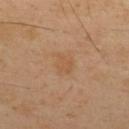Notes:
• lesion size: about 2.5 mm
• subject: male, aged around 50
• imaging modality: total-body-photography crop, ~15 mm field of view
• location: the upper back
• tile lighting: cross-polarized illumination
• image-analysis metrics: a footprint of about 5 mm², an eccentricity of roughly 0.65, and a shape-asymmetry score of about 0.3 (0 = symmetric); a lesion color around L≈54 a*≈19 b*≈35 in CIELAB; a nevus-likeness score of about 0/100